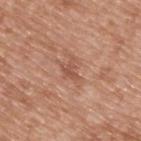Case summary:
– biopsy status: no biopsy performed (imaged during a skin exam)
– body site: the upper back
– diameter: about 3 mm
– patient: male, aged 48–52
– illumination: white-light
– image source: ~15 mm crop, total-body skin-cancer survey